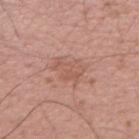Impression: Captured during whole-body skin photography for melanoma surveillance; the lesion was not biopsied. Background: Imaged with white-light lighting. A lesion tile, about 15 mm wide, cut from a 3D total-body photograph. Automated tile analysis of the lesion measured an area of roughly 7 mm², a shape eccentricity near 0.6, and two-axis asymmetry of about 0.35. And it measured about 7 CIELAB-L* units darker than the surrounding skin and a normalized lesion–skin contrast near 5. The software also gave a nevus-likeness score of about 0/100 and lesion-presence confidence of about 100/100. From the right upper arm. A male patient about 40 years old. The recorded lesion diameter is about 3.5 mm.